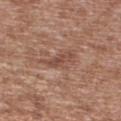Clinical impression:
This lesion was catalogued during total-body skin photography and was not selected for biopsy.
Image and clinical context:
A male subject in their mid- to late 40s. The total-body-photography lesion software estimated a shape eccentricity near 0.9 and a shape-asymmetry score of about 0.35 (0 = symmetric). It also reported a lesion color around L≈49 a*≈21 b*≈27 in CIELAB, a lesion–skin lightness drop of about 8, and a lesion-to-skin contrast of about 6 (normalized; higher = more distinct). The software also gave peripheral color asymmetry of about 1. The lesion is located on the arm. Cropped from a whole-body photographic skin survey; the tile spans about 15 mm.